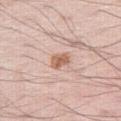Impression:
This lesion was catalogued during total-body skin photography and was not selected for biopsy.
Acquisition and patient details:
Cropped from a total-body skin-imaging series; the visible field is about 15 mm. Captured under white-light illumination. On the right thigh. The patient is a male in their 60s.